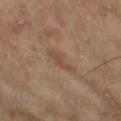Clinical impression:
Imaged during a routine full-body skin examination; the lesion was not biopsied and no histopathology is available.
Acquisition and patient details:
The lesion's longest dimension is about 4 mm. A 15 mm close-up tile from a total-body photography series done for melanoma screening. The tile uses cross-polarized illumination. A female patient, aged approximately 75. Located on the leg.